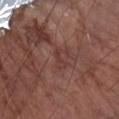Q: Was this lesion biopsied?
A: imaged on a skin check; not biopsied
Q: What are the patient's age and sex?
A: male, approximately 75 years of age
Q: Where on the body is the lesion?
A: the right forearm
Q: How was this image acquired?
A: total-body-photography crop, ~15 mm field of view